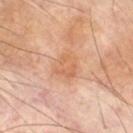Notes:
– follow-up · no biopsy performed (imaged during a skin exam)
– image-analysis metrics · an area of roughly 8.5 mm² and two-axis asymmetry of about 0.25; a mean CIELAB color near L≈62 a*≈22 b*≈35 and a lesion–skin lightness drop of about 7; a border-irregularity index near 2/10 and radial color variation of about 1
– site · the right thigh
– subject · male, aged 68 to 72
– lighting · cross-polarized illumination
– acquisition · total-body-photography crop, ~15 mm field of view
– lesion diameter · ≈3.5 mm